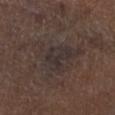This lesion was catalogued during total-body skin photography and was not selected for biopsy. Approximately 6.5 mm at its widest. A male subject, approximately 75 years of age. The lesion is on the left lower leg. A 15 mm close-up tile from a total-body photography series done for melanoma screening. Imaged with white-light lighting.